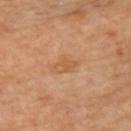No biopsy was performed on this lesion — it was imaged during a full skin examination and was not determined to be concerning. A female subject, approximately 65 years of age. From the upper back. The recorded lesion diameter is about 3 mm. This image is a 15 mm lesion crop taken from a total-body photograph.